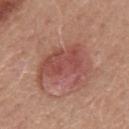{
  "site": "mid back",
  "image": {
    "source": "total-body photography crop",
    "field_of_view_mm": 15
  },
  "patient": {
    "sex": "male",
    "age_approx": 50
  },
  "automated_metrics": {
    "cielab_L": 49,
    "cielab_a": 26,
    "cielab_b": 26,
    "vs_skin_darker_L": 9.0,
    "vs_skin_contrast_norm": 6.5,
    "color_variation_0_10": 3.5,
    "peripheral_color_asymmetry": 1.0,
    "lesion_detection_confidence_0_100": 100
  },
  "lesion_size": {
    "long_diameter_mm_approx": 6.0
  },
  "lighting": "white-light"
}